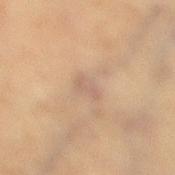Case summary:
- automated metrics — a footprint of about 4 mm², a shape eccentricity near 0.7, and two-axis asymmetry of about 0.25; a border-irregularity rating of about 2.5/10 and a peripheral color-asymmetry measure near 0.5
- site — the right lower leg
- image source — ~15 mm tile from a whole-body skin photo
- patient — female, aged approximately 50
- size — ≈2.5 mm